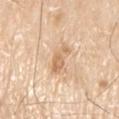Recorded during total-body skin imaging; not selected for excision or biopsy.
Approximately 4 mm at its widest.
Located on the left upper arm.
Captured under white-light illumination.
A 15 mm close-up tile from a total-body photography series done for melanoma screening.
An algorithmic analysis of the crop reported an outline eccentricity of about 0.95 (0 = round, 1 = elongated). It also reported an average lesion color of about L≈68 a*≈18 b*≈36 (CIELAB) and a lesion-to-skin contrast of about 6.5 (normalized; higher = more distinct). It also reported a border-irregularity rating of about 5.5/10, a within-lesion color-variation index near 1.5/10, and a peripheral color-asymmetry measure near 0.5.
A male subject, approximately 80 years of age.